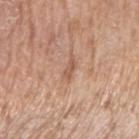{"biopsy_status": "not biopsied; imaged during a skin examination", "automated_metrics": {"cielab_L": 57, "cielab_a": 21, "cielab_b": 31, "vs_skin_darker_L": 8.0, "lesion_detection_confidence_0_100": 95}, "lighting": "white-light", "patient": {"sex": "male", "age_approx": 65}, "site": "right upper arm", "image": {"source": "total-body photography crop", "field_of_view_mm": 15}}